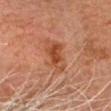image source=15 mm crop, total-body photography | subject=male, roughly 80 years of age | lighting=cross-polarized illumination | anatomic site=the head or neck.